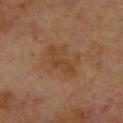Impression: No biopsy was performed on this lesion — it was imaged during a full skin examination and was not determined to be concerning. Background: A roughly 15 mm field-of-view crop from a total-body skin photograph. Located on the right forearm. The tile uses cross-polarized illumination. Automated image analysis of the tile measured a border-irregularity rating of about 3/10, a color-variation rating of about 3/10, and radial color variation of about 1. The recorded lesion diameter is about 5 mm. A female subject, roughly 80 years of age.